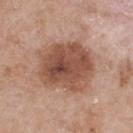Case summary:
• biopsy status · no biopsy performed (imaged during a skin exam)